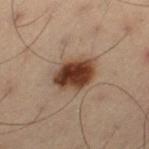Findings:
• follow-up: no biopsy performed (imaged during a skin exam)
• lighting: cross-polarized
• subject: male, about 55 years old
• location: the left thigh
• automated lesion analysis: a lesion color around L≈31 a*≈16 b*≈24 in CIELAB and a normalized lesion–skin contrast near 13.5
• image source: total-body-photography crop, ~15 mm field of view